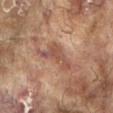Impression: No biopsy was performed on this lesion — it was imaged during a full skin examination and was not determined to be concerning. Image and clinical context: A female subject, aged 78–82. Cropped from a whole-body photographic skin survey; the tile spans about 15 mm. Imaged with cross-polarized lighting. Located on the left arm. The total-body-photography lesion software estimated a mean CIELAB color near L≈45 a*≈20 b*≈25, a lesion–skin lightness drop of about 8, and a lesion-to-skin contrast of about 6.5 (normalized; higher = more distinct). And it measured border irregularity of about 7 on a 0–10 scale and a within-lesion color-variation index near 3.5/10. The analysis additionally found a nevus-likeness score of about 0/100 and a lesion-detection confidence of about 95/100.